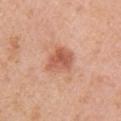| key | value |
|---|---|
| follow-up | total-body-photography surveillance lesion; no biopsy |
| patient | female, aged 38 to 42 |
| tile lighting | white-light illumination |
| lesion size | about 3.5 mm |
| body site | the right upper arm |
| acquisition | 15 mm crop, total-body photography |
| automated lesion analysis | a lesion area of about 8 mm²; a color-variation rating of about 4/10 and radial color variation of about 1.5; an automated nevus-likeness rating near 85 out of 100 and a detector confidence of about 100 out of 100 that the crop contains a lesion |